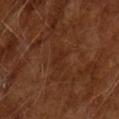{"biopsy_status": "not biopsied; imaged during a skin examination", "automated_metrics": {"cielab_L": 26, "cielab_a": 21, "cielab_b": 28, "vs_skin_darker_L": 4.0, "vs_skin_contrast_norm": 4.5, "border_irregularity_0_10": 8.0, "color_variation_0_10": 0.0, "nevus_likeness_0_100": 0, "lesion_detection_confidence_0_100": 100}, "patient": {"sex": "male", "age_approx": 65}, "image": {"source": "total-body photography crop", "field_of_view_mm": 15}, "lesion_size": {"long_diameter_mm_approx": 2.5}}